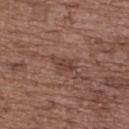No biopsy was performed on this lesion — it was imaged during a full skin examination and was not determined to be concerning. A roughly 15 mm field-of-view crop from a total-body skin photograph. A female subject aged 73 to 77. From the back.